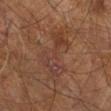  lesion_size:
    long_diameter_mm_approx: 7.0
  site: right leg
  image:
    source: total-body photography crop
    field_of_view_mm: 15
  patient:
    sex: male
    age_approx: 60